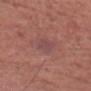Captured during whole-body skin photography for melanoma surveillance; the lesion was not biopsied. The total-body-photography lesion software estimated lesion-presence confidence of about 95/100. A male patient, about 75 years old. A region of skin cropped from a whole-body photographic capture, roughly 15 mm wide. The recorded lesion diameter is about 2.5 mm. The lesion is on the head or neck.